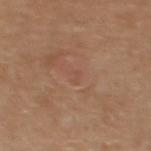| field | value |
|---|---|
| follow-up | no biopsy performed (imaged during a skin exam) |
| TBP lesion metrics | a footprint of about 1.5 mm², a shape eccentricity near 0.3, and two-axis asymmetry of about 0.3; an automated nevus-likeness rating near 0 out of 100 and a lesion-detection confidence of about 100/100 |
| location | the back |
| illumination | white-light |
| diameter | ≈1.5 mm |
| patient | female, about 55 years old |
| image source | total-body-photography crop, ~15 mm field of view |